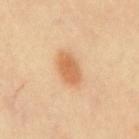Imaged during a routine full-body skin examination; the lesion was not biopsied and no histopathology is available. Cropped from a whole-body photographic skin survey; the tile spans about 15 mm. The subject is a female aged around 40. Located on the mid back.